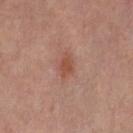Q: Was this lesion biopsied?
A: imaged on a skin check; not biopsied
Q: What is the anatomic site?
A: the right thigh
Q: Who is the patient?
A: female, about 50 years old
Q: What is the imaging modality?
A: ~15 mm tile from a whole-body skin photo